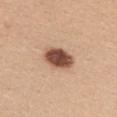Impression:
No biopsy was performed on this lesion — it was imaged during a full skin examination and was not determined to be concerning.
Clinical summary:
The lesion is located on the mid back. Measured at roughly 4.5 mm in maximum diameter. A female patient about 30 years old. The tile uses white-light illumination. This image is a 15 mm lesion crop taken from a total-body photograph.